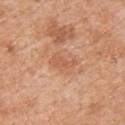{"biopsy_status": "not biopsied; imaged during a skin examination", "lesion_size": {"long_diameter_mm_approx": 3.5}, "automated_metrics": {"area_mm2_approx": 5.0, "eccentricity": 0.9, "shape_asymmetry": 0.4, "vs_skin_darker_L": 8.0, "vs_skin_contrast_norm": 5.0, "border_irregularity_0_10": 5.0}, "lighting": "white-light", "patient": {"sex": "male", "age_approx": 60}, "site": "upper back", "image": {"source": "total-body photography crop", "field_of_view_mm": 15}}